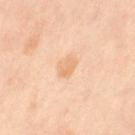Imaged during a routine full-body skin examination; the lesion was not biopsied and no histopathology is available. The lesion is on the left thigh. A female patient aged approximately 50. This image is a 15 mm lesion crop taken from a total-body photograph. Measured at roughly 2.5 mm in maximum diameter.